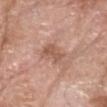Q: Was a biopsy performed?
A: catalogued during a skin exam; not biopsied
Q: Patient demographics?
A: male, approximately 65 years of age
Q: How was the tile lit?
A: white-light illumination
Q: How large is the lesion?
A: ≈3 mm
Q: What did automated image analysis measure?
A: a border-irregularity rating of about 4.5/10 and internal color variation of about 2 on a 0–10 scale; a nevus-likeness score of about 0/100 and a lesion-detection confidence of about 100/100
Q: What is the anatomic site?
A: the head or neck
Q: How was this image acquired?
A: ~15 mm tile from a whole-body skin photo